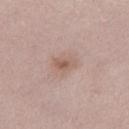{
  "biopsy_status": "not biopsied; imaged during a skin examination",
  "lesion_size": {
    "long_diameter_mm_approx": 3.0
  },
  "image": {
    "source": "total-body photography crop",
    "field_of_view_mm": 15
  },
  "site": "left thigh",
  "automated_metrics": {
    "area_mm2_approx": 4.5,
    "eccentricity": 0.7,
    "cielab_L": 58,
    "cielab_a": 18,
    "cielab_b": 25,
    "vs_skin_darker_L": 8.0,
    "vs_skin_contrast_norm": 6.0,
    "border_irregularity_0_10": 3.0,
    "color_variation_0_10": 3.5,
    "peripheral_color_asymmetry": 1.0
  },
  "patient": {
    "sex": "female",
    "age_approx": 40
  }
}